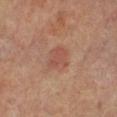Findings:
- follow-up — catalogued during a skin exam; not biopsied
- automated metrics — an area of roughly 6 mm², an eccentricity of roughly 0.45, and a symmetry-axis asymmetry near 0.3; an average lesion color of about L≈49 a*≈23 b*≈27 (CIELAB), about 7 CIELAB-L* units darker than the surrounding skin, and a normalized lesion–skin contrast near 5
- imaging modality — 15 mm crop, total-body photography
- tile lighting — cross-polarized
- diameter — ~3 mm (longest diameter)
- anatomic site — the left leg
- subject — female, in their mid- to late 60s